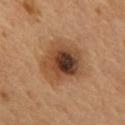Recorded during total-body skin imaging; not selected for excision or biopsy.
On the mid back.
Captured under cross-polarized illumination.
A male patient, aged 53–57.
A close-up tile cropped from a whole-body skin photograph, about 15 mm across.